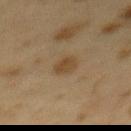Captured during whole-body skin photography for melanoma surveillance; the lesion was not biopsied.
A female patient aged 38 to 42.
A roughly 15 mm field-of-view crop from a total-body skin photograph.
Automated tile analysis of the lesion measured a lesion color around L≈36 a*≈12 b*≈28 in CIELAB, a lesion–skin lightness drop of about 6, and a lesion-to-skin contrast of about 6.5 (normalized; higher = more distinct). The software also gave a nevus-likeness score of about 75/100.
This is a cross-polarized tile.
Located on the back.